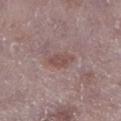<record>
<biopsy_status>not biopsied; imaged during a skin examination</biopsy_status>
<patient>
  <sex>male</sex>
  <age_approx>75</age_approx>
</patient>
<image>
  <source>total-body photography crop</source>
  <field_of_view_mm>15</field_of_view_mm>
</image>
<site>right lower leg</site>
</record>